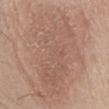Case summary:
– notes: no biopsy performed (imaged during a skin exam)
– size: ≈13.5 mm
– acquisition: ~15 mm tile from a whole-body skin photo
– subject: male, aged approximately 65
– location: the front of the torso